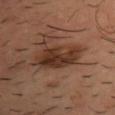The lesion was tiled from a total-body skin photograph and was not biopsied. The tile uses cross-polarized illumination. An algorithmic analysis of the crop reported a border-irregularity index near 4/10, a within-lesion color-variation index near 5/10, and peripheral color asymmetry of about 2. It also reported an automated nevus-likeness rating near 25 out of 100 and lesion-presence confidence of about 100/100. Located on the chest. A roughly 15 mm field-of-view crop from a total-body skin photograph. The lesion's longest dimension is about 6 mm. The patient is a male aged 38–42.